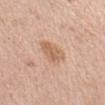{
  "biopsy_status": "not biopsied; imaged during a skin examination",
  "lesion_size": {
    "long_diameter_mm_approx": 3.5
  },
  "image": {
    "source": "total-body photography crop",
    "field_of_view_mm": 15
  },
  "lighting": "white-light",
  "patient": {
    "sex": "female",
    "age_approx": 50
  },
  "site": "left upper arm"
}